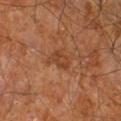Background: The lesion is on the right leg. The total-body-photography lesion software estimated an average lesion color of about L≈40 a*≈23 b*≈33 (CIELAB) and about 7 CIELAB-L* units darker than the surrounding skin. The analysis additionally found border irregularity of about 2.5 on a 0–10 scale, a color-variation rating of about 2/10, and radial color variation of about 1. The software also gave a classifier nevus-likeness of about 0/100. A 15 mm crop from a total-body photograph taken for skin-cancer surveillance. A male patient in their 60s.